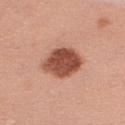| feature | finding |
|---|---|
| workup | imaged on a skin check; not biopsied |
| body site | the right upper arm |
| image | 15 mm crop, total-body photography |
| patient | female, aged 48 to 52 |